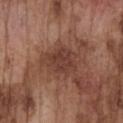This lesion was catalogued during total-body skin photography and was not selected for biopsy.
A lesion tile, about 15 mm wide, cut from a 3D total-body photograph.
Imaged with white-light lighting.
The lesion is on the chest.
The total-body-photography lesion software estimated an average lesion color of about L≈39 a*≈22 b*≈26 (CIELAB), about 9 CIELAB-L* units darker than the surrounding skin, and a normalized border contrast of about 7.5. The analysis additionally found an automated nevus-likeness rating near 5 out of 100 and lesion-presence confidence of about 100/100.
About 4.5 mm across.
A male subject roughly 75 years of age.